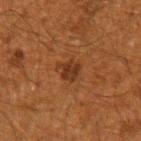Clinical impression: The lesion was photographed on a routine skin check and not biopsied; there is no pathology result. Background: A close-up tile cropped from a whole-body skin photograph, about 15 mm across. From the right upper arm. Captured under cross-polarized illumination. The patient is a male aged 63–67. About 3 mm across. An algorithmic analysis of the crop reported peripheral color asymmetry of about 1. And it measured a nevus-likeness score of about 70/100 and a detector confidence of about 100 out of 100 that the crop contains a lesion.